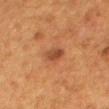Q: Was a biopsy performed?
A: total-body-photography surveillance lesion; no biopsy
Q: Who is the patient?
A: female, aged around 50
Q: How was this image acquired?
A: 15 mm crop, total-body photography
Q: How was the tile lit?
A: cross-polarized
Q: How large is the lesion?
A: ~3 mm (longest diameter)
Q: What is the anatomic site?
A: the mid back